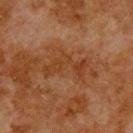workup: catalogued during a skin exam; not biopsied | automated metrics: a lesion area of about 8 mm², an eccentricity of roughly 0.9, and two-axis asymmetry of about 0.6; a lesion color around L≈30 a*≈19 b*≈29 in CIELAB, about 5 CIELAB-L* units darker than the surrounding skin, and a lesion-to-skin contrast of about 6 (normalized; higher = more distinct); an automated nevus-likeness rating near 0 out of 100 and lesion-presence confidence of about 100/100 | tile lighting: cross-polarized illumination | subject: male, in their 80s | anatomic site: the upper back | lesion diameter: about 5 mm | acquisition: total-body-photography crop, ~15 mm field of view.